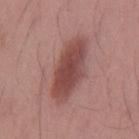This lesion was catalogued during total-body skin photography and was not selected for biopsy. From the mid back. The subject is a male in their mid- to late 30s. The total-body-photography lesion software estimated a lesion area of about 18 mm² and a shape-asymmetry score of about 0.15 (0 = symmetric). It also reported an average lesion color of about L≈46 a*≈24 b*≈22 (CIELAB), a lesion–skin lightness drop of about 12, and a normalized border contrast of about 9. The analysis additionally found border irregularity of about 2 on a 0–10 scale, a color-variation rating of about 3.5/10, and a peripheral color-asymmetry measure near 1. It also reported an automated nevus-likeness rating near 90 out of 100 and lesion-presence confidence of about 100/100. Imaged with white-light lighting. Approximately 7 mm at its widest. A close-up tile cropped from a whole-body skin photograph, about 15 mm across.